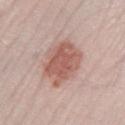{
  "lighting": "white-light",
  "image": {
    "source": "total-body photography crop",
    "field_of_view_mm": 15
  },
  "patient": {
    "sex": "female",
    "age_approx": 40
  },
  "automated_metrics": {
    "cielab_L": 57,
    "cielab_a": 22,
    "cielab_b": 25,
    "vs_skin_darker_L": 12.0,
    "border_irregularity_0_10": 2.5,
    "color_variation_0_10": 3.0,
    "nevus_likeness_0_100": 95,
    "lesion_detection_confidence_0_100": 100
  },
  "site": "left lower leg",
  "lesion_size": {
    "long_diameter_mm_approx": 5.5
  }
}